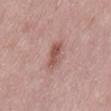Clinical impression: Imaged during a routine full-body skin examination; the lesion was not biopsied and no histopathology is available. Context: A female subject aged around 40. Cropped from a whole-body photographic skin survey; the tile spans about 15 mm. From the back.